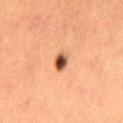  biopsy_status: not biopsied; imaged during a skin examination
  automated_metrics:
    cielab_L: 41
    cielab_a: 22
    cielab_b: 29
    vs_skin_darker_L: 17.0
    vs_skin_contrast_norm: 13.0
  image:
    source: total-body photography crop
    field_of_view_mm: 15
  patient:
    sex: female
    age_approx: 55
  lesion_size:
    long_diameter_mm_approx: 2.5
  lighting: cross-polarized
  site: left thigh